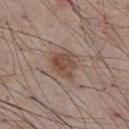| feature | finding |
|---|---|
| follow-up | imaged on a skin check; not biopsied |
| subject | male, approximately 55 years of age |
| lighting | white-light |
| image source | 15 mm crop, total-body photography |
| automated lesion analysis | a lesion area of about 9 mm², an outline eccentricity of about 0.5 (0 = round, 1 = elongated), and a symmetry-axis asymmetry near 0.3; a lesion color around L≈48 a*≈18 b*≈26 in CIELAB and a lesion-to-skin contrast of about 8 (normalized; higher = more distinct); border irregularity of about 3.5 on a 0–10 scale, a color-variation rating of about 4/10, and radial color variation of about 1.5 |
| lesion size | ~4 mm (longest diameter) |
| anatomic site | the abdomen |